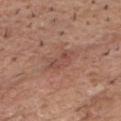{
  "biopsy_status": "not biopsied; imaged during a skin examination",
  "image": {
    "source": "total-body photography crop",
    "field_of_view_mm": 15
  },
  "site": "front of the torso",
  "patient": {
    "sex": "male",
    "age_approx": 80
  },
  "automated_metrics": {
    "area_mm2_approx": 5.5,
    "eccentricity": 0.85,
    "shape_asymmetry": 0.35,
    "cielab_L": 48,
    "cielab_a": 22,
    "cielab_b": 26,
    "vs_skin_darker_L": 7.0,
    "vs_skin_contrast_norm": 5.5,
    "color_variation_0_10": 3.5,
    "peripheral_color_asymmetry": 1.5,
    "nevus_likeness_0_100": 0,
    "lesion_detection_confidence_0_100": 100
  },
  "lighting": "white-light"
}